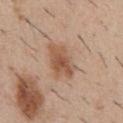Clinical impression: The lesion was photographed on a routine skin check and not biopsied; there is no pathology result. Clinical summary: The recorded lesion diameter is about 5 mm. From the front of the torso. This is a white-light tile. A male subject, roughly 30 years of age. A 15 mm crop from a total-body photograph taken for skin-cancer surveillance. Automated image analysis of the tile measured an area of roughly 10 mm², a shape eccentricity near 0.8, and a symmetry-axis asymmetry near 0.25. The analysis additionally found a mean CIELAB color near L≈54 a*≈20 b*≈31 and a normalized border contrast of about 7.5. It also reported internal color variation of about 4 on a 0–10 scale and peripheral color asymmetry of about 1.